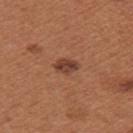Notes:
• tile lighting — white-light
• location — the left upper arm
• subject — female, in their 30s
• size — ~3 mm (longest diameter)
• acquisition — total-body-photography crop, ~15 mm field of view